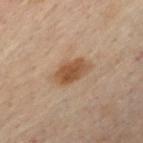Part of a total-body skin-imaging series; this lesion was reviewed on a skin check and was not flagged for biopsy. Captured under cross-polarized illumination. Longest diameter approximately 4 mm. A lesion tile, about 15 mm wide, cut from a 3D total-body photograph. A male subject, aged 48–52. The lesion is located on the chest.